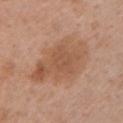Assessment:
No biopsy was performed on this lesion — it was imaged during a full skin examination and was not determined to be concerning.
Clinical summary:
Captured under white-light illumination. A 15 mm crop from a total-body photograph taken for skin-cancer surveillance. On the left upper arm. A female patient about 40 years old. Approximately 8.5 mm at its widest.